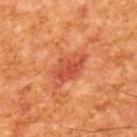Imaged during a routine full-body skin examination; the lesion was not biopsied and no histopathology is available. Cropped from a total-body skin-imaging series; the visible field is about 15 mm. The tile uses cross-polarized illumination. Longest diameter approximately 5 mm. The subject is a male about 65 years old.